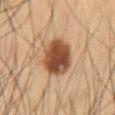The lesion was tiled from a total-body skin photograph and was not biopsied.
The lesion is on the front of the torso.
A lesion tile, about 15 mm wide, cut from a 3D total-body photograph.
A male patient aged 53 to 57.